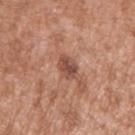<case>
<biopsy_status>not biopsied; imaged during a skin examination</biopsy_status>
<site>right upper arm</site>
<image>
  <source>total-body photography crop</source>
  <field_of_view_mm>15</field_of_view_mm>
</image>
<patient>
  <sex>male</sex>
  <age_approx>45</age_approx>
</patient>
</case>